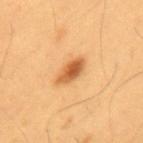This lesion was catalogued during total-body skin photography and was not selected for biopsy. A male subject roughly 55 years of age. The lesion is on the mid back. Captured under cross-polarized illumination. A 15 mm close-up extracted from a 3D total-body photography capture.